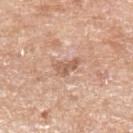follow-up: total-body-photography surveillance lesion; no biopsy
acquisition: total-body-photography crop, ~15 mm field of view
lighting: white-light illumination
TBP lesion metrics: a lesion area of about 4 mm², an outline eccentricity of about 0.85 (0 = round, 1 = elongated), and two-axis asymmetry of about 0.4; a within-lesion color-variation index near 1.5/10 and a peripheral color-asymmetry measure near 0.5; lesion-presence confidence of about 100/100
patient: female, in their mid-70s
location: the left forearm
lesion diameter: ~3 mm (longest diameter)